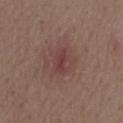Part of a total-body skin-imaging series; this lesion was reviewed on a skin check and was not flagged for biopsy. Cropped from a whole-body photographic skin survey; the tile spans about 15 mm. Approximately 3 mm at its widest. Automated tile analysis of the lesion measured a lesion area of about 5 mm² and a shape eccentricity near 0.75. It also reported a lesion color around L≈40 a*≈23 b*≈19 in CIELAB and a normalized lesion–skin contrast near 6. The analysis additionally found border irregularity of about 3 on a 0–10 scale, internal color variation of about 1.5 on a 0–10 scale, and radial color variation of about 0.5. It also reported lesion-presence confidence of about 100/100. The tile uses white-light illumination. The lesion is located on the back. A male patient, roughly 70 years of age.